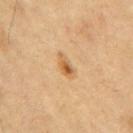notes: total-body-photography surveillance lesion; no biopsy
diameter: about 3 mm
image: ~15 mm tile from a whole-body skin photo
illumination: cross-polarized
subject: male, aged 68 to 72
location: the right upper arm